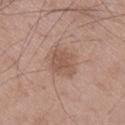Clinical impression:
This lesion was catalogued during total-body skin photography and was not selected for biopsy.
Image and clinical context:
About 3.5 mm across. A 15 mm crop from a total-body photograph taken for skin-cancer surveillance. The lesion is on the right lower leg. The patient is a male aged approximately 50. Captured under white-light illumination.